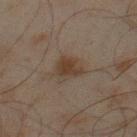Context:
Longest diameter approximately 3.5 mm. The lesion is on the leg. This image is a 15 mm lesion crop taken from a total-body photograph. The total-body-photography lesion software estimated an outline eccentricity of about 0.8 (0 = round, 1 = elongated) and a symmetry-axis asymmetry near 0.35. The software also gave about 7 CIELAB-L* units darker than the surrounding skin and a normalized border contrast of about 8.5. It also reported a border-irregularity index near 3.5/10, a within-lesion color-variation index near 2/10, and radial color variation of about 0.5. The software also gave a classifier nevus-likeness of about 55/100 and a detector confidence of about 100 out of 100 that the crop contains a lesion. Captured under cross-polarized illumination. A male patient, aged around 45.